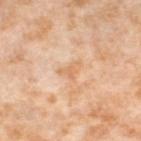The lesion was photographed on a routine skin check and not biopsied; there is no pathology result. Cropped from a total-body skin-imaging series; the visible field is about 15 mm. A female patient, about 55 years old. Located on the left thigh.